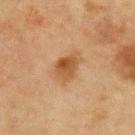{"biopsy_status": "not biopsied; imaged during a skin examination", "site": "chest", "lesion_size": {"long_diameter_mm_approx": 3.5}, "patient": {"sex": "male", "age_approx": 65}, "image": {"source": "total-body photography crop", "field_of_view_mm": 15}, "lighting": "cross-polarized"}